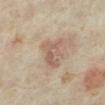Q: Was this lesion biopsied?
A: catalogued during a skin exam; not biopsied
Q: Patient demographics?
A: female, approximately 35 years of age
Q: How was this image acquired?
A: total-body-photography crop, ~15 mm field of view
Q: What is the anatomic site?
A: the left lower leg
Q: Lesion size?
A: about 4 mm